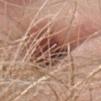{"biopsy_status": "not biopsied; imaged during a skin examination", "automated_metrics": {"area_mm2_approx": 19.0, "eccentricity": 0.7, "shape_asymmetry": 0.15, "cielab_L": 47, "cielab_a": 20, "cielab_b": 26, "vs_skin_darker_L": 14.0, "vs_skin_contrast_norm": 10.5, "border_irregularity_0_10": 2.0, "color_variation_0_10": 9.5}, "patient": {"sex": "female", "age_approx": 65}, "lighting": "white-light", "image": {"source": "total-body photography crop", "field_of_view_mm": 15}, "site": "head or neck", "lesion_size": {"long_diameter_mm_approx": 6.0}}